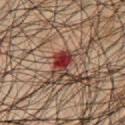Clinical impression:
No biopsy was performed on this lesion — it was imaged during a full skin examination and was not determined to be concerning.
Image and clinical context:
The lesion is located on the front of the torso. A roughly 15 mm field-of-view crop from a total-body skin photograph. A male subject in their mid-60s.